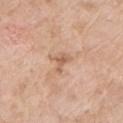Clinical impression: Imaged during a routine full-body skin examination; the lesion was not biopsied and no histopathology is available. Background: A female patient, aged 73–77. Imaged with white-light lighting. From the left upper arm. An algorithmic analysis of the crop reported a border-irregularity rating of about 6.5/10 and peripheral color asymmetry of about 0. The lesion's longest dimension is about 2.5 mm. A roughly 15 mm field-of-view crop from a total-body skin photograph.